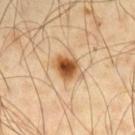Clinical impression:
Captured during whole-body skin photography for melanoma surveillance; the lesion was not biopsied.
Acquisition and patient details:
A male patient in their mid-60s. Measured at roughly 4 mm in maximum diameter. A 15 mm close-up extracted from a 3D total-body photography capture. From the arm. The tile uses cross-polarized illumination. Automated image analysis of the tile measured an area of roughly 8.5 mm², a shape eccentricity near 0.65, and a shape-asymmetry score of about 0.2 (0 = symmetric). The software also gave an average lesion color of about L≈57 a*≈21 b*≈39 (CIELAB), roughly 16 lightness units darker than nearby skin, and a normalized lesion–skin contrast near 10.5. And it measured a classifier nevus-likeness of about 100/100.